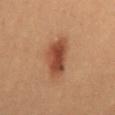Assessment:
No biopsy was performed on this lesion — it was imaged during a full skin examination and was not determined to be concerning.
Background:
A close-up tile cropped from a whole-body skin photograph, about 15 mm across. An algorithmic analysis of the crop reported a shape eccentricity near 0.8 and a symmetry-axis asymmetry near 0.25. The software also gave border irregularity of about 2.5 on a 0–10 scale, internal color variation of about 3.5 on a 0–10 scale, and a peripheral color-asymmetry measure near 1. It also reported a classifier nevus-likeness of about 100/100. This is a cross-polarized tile. Measured at roughly 4.5 mm in maximum diameter. A female patient aged 18–22. Located on the mid back.